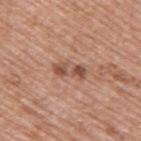The lesion was photographed on a routine skin check and not biopsied; there is no pathology result. The subject is a male approximately 70 years of age. This is a white-light tile. The lesion is located on the back. A region of skin cropped from a whole-body photographic capture, roughly 15 mm wide.